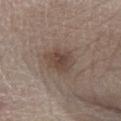Findings:
– follow-up: imaged on a skin check; not biopsied
– acquisition: 15 mm crop, total-body photography
– lesion size: ≈4 mm
– patient: male, roughly 75 years of age
– automated lesion analysis: a footprint of about 9.5 mm², an eccentricity of roughly 0.65, and two-axis asymmetry of about 0.2; border irregularity of about 2 on a 0–10 scale and internal color variation of about 3.5 on a 0–10 scale
– site: the left lower leg
– lighting: white-light illumination